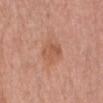The lesion is on the abdomen. A male subject, roughly 65 years of age. About 3.5 mm across. A lesion tile, about 15 mm wide, cut from a 3D total-body photograph. The total-body-photography lesion software estimated two-axis asymmetry of about 0.2. The analysis additionally found a lesion–skin lightness drop of about 7 and a normalized lesion–skin contrast near 5.5. The software also gave a border-irregularity index near 2.5/10, a within-lesion color-variation index near 3.5/10, and radial color variation of about 1. And it measured a nevus-likeness score of about 5/100 and lesion-presence confidence of about 100/100.